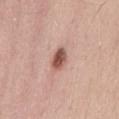{"biopsy_status": "not biopsied; imaged during a skin examination", "image": {"source": "total-body photography crop", "field_of_view_mm": 15}, "site": "lower back", "patient": {"sex": "male", "age_approx": 25}}